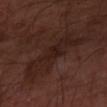notes — no biopsy performed (imaged during a skin exam); image source — 15 mm crop, total-body photography; anatomic site — the left forearm.